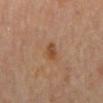Captured during whole-body skin photography for melanoma surveillance; the lesion was not biopsied. The subject is a male aged 63–67. On the mid back. Imaged with cross-polarized lighting. The lesion's longest dimension is about 3 mm. Cropped from a whole-body photographic skin survey; the tile spans about 15 mm.